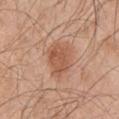Case summary:
– biopsy status — catalogued during a skin exam; not biopsied
– lesion diameter — about 4 mm
– subject — male, in their mid-40s
– acquisition — total-body-photography crop, ~15 mm field of view
– site — the right upper arm
– image-analysis metrics — a footprint of about 11 mm², a shape eccentricity near 0.55, and a shape-asymmetry score of about 0.2 (0 = symmetric)